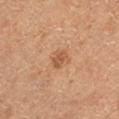<case>
  <biopsy_status>not biopsied; imaged during a skin examination</biopsy_status>
  <patient>
    <sex>male</sex>
    <age_approx>60</age_approx>
  </patient>
  <lighting>cross-polarized</lighting>
  <lesion_size>
    <long_diameter_mm_approx>2.5</long_diameter_mm_approx>
  </lesion_size>
  <image>
    <source>total-body photography crop</source>
    <field_of_view_mm>15</field_of_view_mm>
  </image>
  <site>left lower leg</site>
</case>